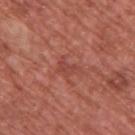Impression:
The lesion was tiled from a total-body skin photograph and was not biopsied.
Context:
The subject is a male approximately 55 years of age. Located on the upper back. A 15 mm crop from a total-body photograph taken for skin-cancer surveillance.